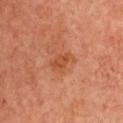This lesion was catalogued during total-body skin photography and was not selected for biopsy.
The recorded lesion diameter is about 3 mm.
The lesion is on the chest.
A female subject, in their mid- to late 60s.
This image is a 15 mm lesion crop taken from a total-body photograph.
Automated image analysis of the tile measured a lesion color around L≈47 a*≈27 b*≈36 in CIELAB and a normalized border contrast of about 6.5. It also reported border irregularity of about 3 on a 0–10 scale and a color-variation rating of about 2/10.
Captured under cross-polarized illumination.